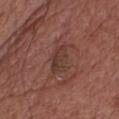Imaged during a routine full-body skin examination; the lesion was not biopsied and no histopathology is available. Cropped from a total-body skin-imaging series; the visible field is about 15 mm. About 4 mm across. A male subject aged approximately 65. On the chest. Automated tile analysis of the lesion measured a lesion area of about 7 mm² and a symmetry-axis asymmetry near 0.25. Imaged with white-light lighting.